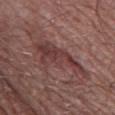Clinical impression:
Imaged during a routine full-body skin examination; the lesion was not biopsied and no histopathology is available.
Background:
Cropped from a whole-body photographic skin survey; the tile spans about 15 mm. The recorded lesion diameter is about 7.5 mm. A male patient, in their mid-60s. An algorithmic analysis of the crop reported a mean CIELAB color near L≈38 a*≈22 b*≈20, roughly 9 lightness units darker than nearby skin, and a normalized lesion–skin contrast near 7.5. And it measured border irregularity of about 7 on a 0–10 scale, a color-variation rating of about 4.5/10, and a peripheral color-asymmetry measure near 1.5. And it measured an automated nevus-likeness rating near 5 out of 100 and a lesion-detection confidence of about 75/100. The lesion is located on the leg. Imaged with white-light lighting.